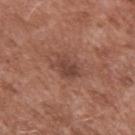| key | value |
|---|---|
| TBP lesion metrics | a border-irregularity rating of about 4/10, a within-lesion color-variation index near 2/10, and a peripheral color-asymmetry measure near 0.5; lesion-presence confidence of about 100/100 |
| subject | male, aged approximately 55 |
| lesion size | ~3 mm (longest diameter) |
| anatomic site | the left upper arm |
| imaging modality | 15 mm crop, total-body photography |
| lighting | white-light |